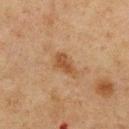workup — no biopsy performed (imaged during a skin exam)
imaging modality — ~15 mm tile from a whole-body skin photo
size — about 3.5 mm
illumination — cross-polarized
image-analysis metrics — a mean CIELAB color near L≈41 a*≈17 b*≈31 and a normalized lesion–skin contrast near 7; border irregularity of about 3.5 on a 0–10 scale, internal color variation of about 2 on a 0–10 scale, and radial color variation of about 1
body site — the front of the torso
subject — male, approximately 75 years of age